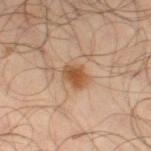No biopsy was performed on this lesion — it was imaged during a full skin examination and was not determined to be concerning. From the left thigh. Automated tile analysis of the lesion measured a lesion–skin lightness drop of about 11 and a normalized lesion–skin contrast near 9.5. And it measured a lesion-detection confidence of about 100/100. Approximately 2.5 mm at its widest. This is a cross-polarized tile. The patient is a male aged 63–67. A roughly 15 mm field-of-view crop from a total-body skin photograph.